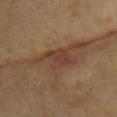Q: How was this image acquired?
A: 15 mm crop, total-body photography
Q: Patient demographics?
A: female, aged approximately 75
Q: Lesion location?
A: the front of the torso